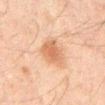Background:
A region of skin cropped from a whole-body photographic capture, roughly 15 mm wide. From the mid back. A male subject approximately 45 years of age. Automated image analysis of the tile measured a lesion area of about 9 mm² and a symmetry-axis asymmetry near 0.2. And it measured a border-irregularity index near 2.5/10 and a color-variation rating of about 3/10. The tile uses cross-polarized illumination.